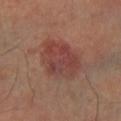Clinical impression:
No biopsy was performed on this lesion — it was imaged during a full skin examination and was not determined to be concerning.
Background:
Cropped from a whole-body photographic skin survey; the tile spans about 15 mm. The patient is aged 63–67. Measured at roughly 6 mm in maximum diameter. On the left lower leg.